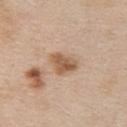Notes:
• biopsy status: imaged on a skin check; not biopsied
• subject: female, aged around 45
• tile lighting: white-light
• body site: the chest
• size: about 3.5 mm
• automated lesion analysis: a footprint of about 7 mm² and an outline eccentricity of about 0.7 (0 = round, 1 = elongated); a mean CIELAB color near L≈57 a*≈19 b*≈33, about 12 CIELAB-L* units darker than the surrounding skin, and a normalized border contrast of about 8.5; border irregularity of about 2.5 on a 0–10 scale and a within-lesion color-variation index near 4.5/10; an automated nevus-likeness rating near 70 out of 100 and a lesion-detection confidence of about 100/100
• imaging modality: total-body-photography crop, ~15 mm field of view